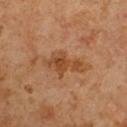Clinical impression:
The lesion was photographed on a routine skin check and not biopsied; there is no pathology result.
Context:
The subject is a male aged 63 to 67. Longest diameter approximately 5 mm. A 15 mm close-up tile from a total-body photography series done for melanoma screening. The tile uses cross-polarized illumination.